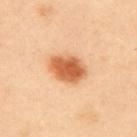The lesion is located on the upper back.
The subject is a female about 40 years old.
Cropped from a total-body skin-imaging series; the visible field is about 15 mm.